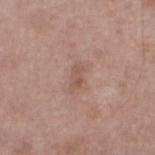{"biopsy_status": "not biopsied; imaged during a skin examination", "site": "leg", "patient": {"sex": "male", "age_approx": 65}, "image": {"source": "total-body photography crop", "field_of_view_mm": 15}, "lighting": "white-light", "automated_metrics": {"area_mm2_approx": 3.5, "eccentricity": 0.85, "shape_asymmetry": 0.35, "color_variation_0_10": 1.0, "peripheral_color_asymmetry": 0.0, "nevus_likeness_0_100": 0, "lesion_detection_confidence_0_100": 100}}